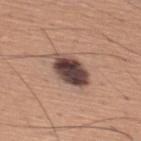The recorded lesion diameter is about 5 mm. The tile uses white-light illumination. A 15 mm crop from a total-body photograph taken for skin-cancer surveillance. The subject is a male approximately 45 years of age. From the back.